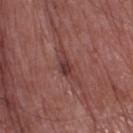{
  "biopsy_status": "not biopsied; imaged during a skin examination",
  "lesion_size": {
    "long_diameter_mm_approx": 5.0
  },
  "image": {
    "source": "total-body photography crop",
    "field_of_view_mm": 15
  },
  "site": "left thigh",
  "patient": {
    "sex": "male",
    "age_approx": "85+"
  },
  "lighting": "white-light"
}